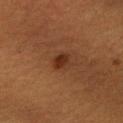The lesion was photographed on a routine skin check and not biopsied; there is no pathology result.
A close-up tile cropped from a whole-body skin photograph, about 15 mm across.
About 3 mm across.
Located on the arm.
The patient is a female aged 53–57.
Automated image analysis of the tile measured a footprint of about 4 mm², an outline eccentricity of about 0.8 (0 = round, 1 = elongated), and a shape-asymmetry score of about 0.2 (0 = symmetric). It also reported a classifier nevus-likeness of about 95/100 and a lesion-detection confidence of about 100/100.
Captured under cross-polarized illumination.